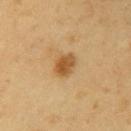The lesion was photographed on a routine skin check and not biopsied; there is no pathology result.